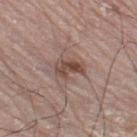Case summary:
– biopsy status: total-body-photography surveillance lesion; no biopsy
– illumination: white-light illumination
– lesion size: ~3.5 mm (longest diameter)
– patient: male, about 70 years old
– image source: total-body-photography crop, ~15 mm field of view
– body site: the leg